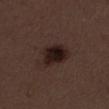This lesion was catalogued during total-body skin photography and was not selected for biopsy. This image is a 15 mm lesion crop taken from a total-body photograph. A female subject in their 50s. Located on the left thigh. Approximately 4 mm at its widest. The tile uses white-light illumination.